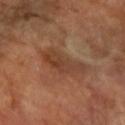Clinical impression:
Recorded during total-body skin imaging; not selected for excision or biopsy.
Background:
A female patient, about 60 years old. Cropped from a whole-body photographic skin survey; the tile spans about 15 mm. Measured at roughly 5.5 mm in maximum diameter. The tile uses cross-polarized illumination. Automated tile analysis of the lesion measured an average lesion color of about L≈41 a*≈21 b*≈31 (CIELAB) and roughly 8 lightness units darker than nearby skin. It also reported a classifier nevus-likeness of about 0/100 and lesion-presence confidence of about 100/100. The lesion is on the left arm.